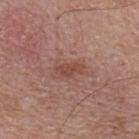| feature | finding |
|---|---|
| image | total-body-photography crop, ~15 mm field of view |
| diameter | ≈3.5 mm |
| illumination | white-light illumination |
| subject | male, aged 63–67 |
| site | the upper back |